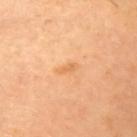{
  "biopsy_status": "not biopsied; imaged during a skin examination",
  "image": {
    "source": "total-body photography crop",
    "field_of_view_mm": 15
  },
  "site": "right upper arm",
  "lighting": "cross-polarized",
  "lesion_size": {
    "long_diameter_mm_approx": 2.5
  },
  "patient": {
    "sex": "female",
    "age_approx": 70
  }
}